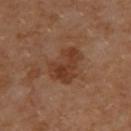No biopsy was performed on this lesion — it was imaged during a full skin examination and was not determined to be concerning.
The total-body-photography lesion software estimated a footprint of about 12 mm² and two-axis asymmetry of about 0.3.
This is a cross-polarized tile.
Measured at roughly 4.5 mm in maximum diameter.
A 15 mm close-up extracted from a 3D total-body photography capture.
The subject is a female roughly 60 years of age.
On the upper back.